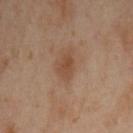Clinical impression:
Imaged during a routine full-body skin examination; the lesion was not biopsied and no histopathology is available.
Context:
A 15 mm close-up tile from a total-body photography series done for melanoma screening. A female subject, aged around 40. The lesion is located on the left upper arm. Imaged with cross-polarized lighting.